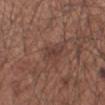Clinical impression:
Captured during whole-body skin photography for melanoma surveillance; the lesion was not biopsied.
Image and clinical context:
A male patient in their mid- to late 50s. About 3 mm across. Automated image analysis of the tile measured an eccentricity of roughly 0.85 and a shape-asymmetry score of about 0.25 (0 = symmetric). The analysis additionally found a lesion color around L≈39 a*≈19 b*≈23 in CIELAB, about 7 CIELAB-L* units darker than the surrounding skin, and a normalized border contrast of about 6. The software also gave a border-irregularity rating of about 2.5/10 and peripheral color asymmetry of about 0.5. It also reported a classifier nevus-likeness of about 5/100 and lesion-presence confidence of about 65/100. This image is a 15 mm lesion crop taken from a total-body photograph. Located on the left upper arm.